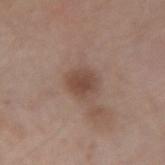Clinical impression: Imaged during a routine full-body skin examination; the lesion was not biopsied and no histopathology is available. Acquisition and patient details: A male patient, aged 58–62. The lesion is on the left forearm. Measured at roughly 3 mm in maximum diameter. Imaged with white-light lighting. Cropped from a total-body skin-imaging series; the visible field is about 15 mm.